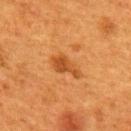A female patient in their 40s. The recorded lesion diameter is about 4 mm. The total-body-photography lesion software estimated a footprint of about 6 mm² and a shape eccentricity near 0.85. It also reported an average lesion color of about L≈42 a*≈25 b*≈39 (CIELAB), a lesion–skin lightness drop of about 9, and a lesion-to-skin contrast of about 7 (normalized; higher = more distinct). And it measured border irregularity of about 4.5 on a 0–10 scale, a within-lesion color-variation index near 2.5/10, and a peripheral color-asymmetry measure near 0.5. The software also gave a classifier nevus-likeness of about 90/100. A 15 mm close-up extracted from a 3D total-body photography capture. This is a cross-polarized tile. Located on the upper back.